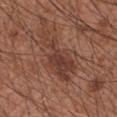Case summary:
• follow-up — catalogued during a skin exam; not biopsied
• body site — the right forearm
• subject — male, aged around 30
• image — total-body-photography crop, ~15 mm field of view